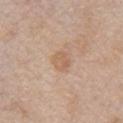{"biopsy_status": "not biopsied; imaged during a skin examination", "lesion_size": {"long_diameter_mm_approx": 2.5}, "image": {"source": "total-body photography crop", "field_of_view_mm": 15}, "site": "arm", "patient": {"sex": "female", "age_approx": 65}, "lighting": "white-light"}